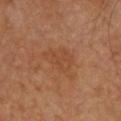Recorded during total-body skin imaging; not selected for excision or biopsy.
A 15 mm crop from a total-body photograph taken for skin-cancer surveillance.
Automated tile analysis of the lesion measured a within-lesion color-variation index near 3/10 and radial color variation of about 1.
The tile uses cross-polarized illumination.
The patient is a male about 60 years old.
Located on the back.
Measured at roughly 5 mm in maximum diameter.